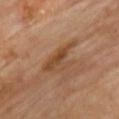Assessment: The lesion was tiled from a total-body skin photograph and was not biopsied. Context: The lesion's longest dimension is about 5.5 mm. Automated image analysis of the tile measured a lesion area of about 9 mm² and a symmetry-axis asymmetry near 0.5. It also reported a border-irregularity rating of about 6.5/10, internal color variation of about 5.5 on a 0–10 scale, and radial color variation of about 2. It also reported a classifier nevus-likeness of about 0/100 and a detector confidence of about 100 out of 100 that the crop contains a lesion. Cropped from a whole-body photographic skin survey; the tile spans about 15 mm. The lesion is located on the chest. A male subject, aged around 70.